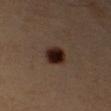{
  "biopsy_status": "not biopsied; imaged during a skin examination",
  "site": "right upper arm",
  "lighting": "cross-polarized",
  "patient": {
    "sex": "male",
    "age_approx": 55
  },
  "image": {
    "source": "total-body photography crop",
    "field_of_view_mm": 15
  },
  "lesion_size": {
    "long_diameter_mm_approx": 3.0
  },
  "automated_metrics": {
    "area_mm2_approx": 6.0,
    "eccentricity": 0.7,
    "shape_asymmetry": 0.2,
    "border_irregularity_0_10": 1.5,
    "color_variation_0_10": 4.5,
    "peripheral_color_asymmetry": 1.0,
    "lesion_detection_confidence_0_100": 100
  }
}